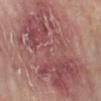| feature | finding |
|---|---|
| biopsy status | catalogued during a skin exam; not biopsied |
| tile lighting | cross-polarized illumination |
| lesion diameter | ≈12.5 mm |
| location | the right lower leg |
| acquisition | total-body-photography crop, ~15 mm field of view |
| TBP lesion metrics | border irregularity of about 5.5 on a 0–10 scale and a peripheral color-asymmetry measure near 2.5; a nevus-likeness score of about 0/100 and a detector confidence of about 100 out of 100 that the crop contains a lesion |
| patient | female, in their mid-70s |